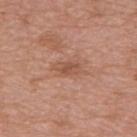Assessment:
The lesion was tiled from a total-body skin photograph and was not biopsied.
Background:
A female patient aged around 75. Cropped from a total-body skin-imaging series; the visible field is about 15 mm. The lesion is located on the upper back. The lesion-visualizer software estimated an average lesion color of about L≈52 a*≈23 b*≈30 (CIELAB), about 8 CIELAB-L* units darker than the surrounding skin, and a lesion-to-skin contrast of about 6 (normalized; higher = more distinct).